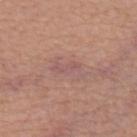Acquisition and patient details:
From the front of the torso. The subject is a male approximately 65 years of age. This is a white-light tile. The lesion-visualizer software estimated a mean CIELAB color near L≈55 a*≈21 b*≈22 and roughly 5 lightness units darker than nearby skin. The analysis additionally found border irregularity of about 4.5 on a 0–10 scale, a within-lesion color-variation index near 1.5/10, and peripheral color asymmetry of about 0.5. The recorded lesion diameter is about 4 mm. A 15 mm close-up tile from a total-body photography series done for melanoma screening.